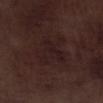<case>
  <biopsy_status>not biopsied; imaged during a skin examination</biopsy_status>
  <lighting>white-light</lighting>
  <image>
    <source>total-body photography crop</source>
    <field_of_view_mm>15</field_of_view_mm>
  </image>
  <patient>
    <sex>male</sex>
    <age_approx>70</age_approx>
  </patient>
  <site>left lower leg</site>
</case>